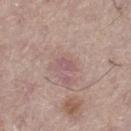Assessment:
No biopsy was performed on this lesion — it was imaged during a full skin examination and was not determined to be concerning.
Acquisition and patient details:
From the left thigh. The tile uses white-light illumination. A 15 mm close-up extracted from a 3D total-body photography capture. Measured at roughly 2.5 mm in maximum diameter. A male subject aged approximately 55.